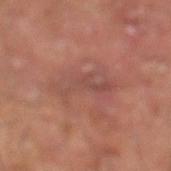biopsy status: no biopsy performed (imaged during a skin exam) | imaging modality: ~15 mm crop, total-body skin-cancer survey | patient: male, aged 68 to 72 | tile lighting: cross-polarized | TBP lesion metrics: an eccentricity of roughly 0.95 and two-axis asymmetry of about 0.4; a mean CIELAB color near L≈47 a*≈23 b*≈24; a classifier nevus-likeness of about 0/100 and lesion-presence confidence of about 90/100 | diameter: ~6.5 mm (longest diameter) | anatomic site: the left lower leg.